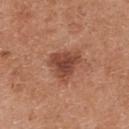biopsy status — no biopsy performed (imaged during a skin exam); subject — female, approximately 40 years of age; anatomic site — the upper back; imaging modality — 15 mm crop, total-body photography.